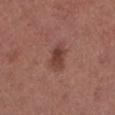Q: Was a biopsy performed?
A: total-body-photography surveillance lesion; no biopsy
Q: Illumination type?
A: white-light illumination
Q: How was this image acquired?
A: ~15 mm tile from a whole-body skin photo
Q: Automated lesion metrics?
A: about 10 CIELAB-L* units darker than the surrounding skin and a normalized lesion–skin contrast near 8; border irregularity of about 1.5 on a 0–10 scale, a color-variation rating of about 5/10, and radial color variation of about 2
Q: Patient demographics?
A: female, aged approximately 65
Q: Where on the body is the lesion?
A: the left lower leg